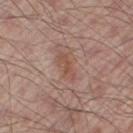image source: total-body-photography crop, ~15 mm field of view
automated metrics: a footprint of about 5 mm² and an eccentricity of roughly 0.9; a mean CIELAB color near L≈51 a*≈21 b*≈27, about 7 CIELAB-L* units darker than the surrounding skin, and a lesion-to-skin contrast of about 5.5 (normalized; higher = more distinct); an automated nevus-likeness rating near 15 out of 100 and lesion-presence confidence of about 100/100
size: ~3.5 mm (longest diameter)
lighting: white-light illumination
location: the right thigh
subject: male, aged 53–57